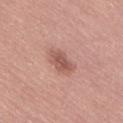acquisition: ~15 mm crop, total-body skin-cancer survey; subject: male, roughly 50 years of age; location: the lower back.